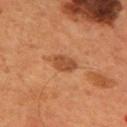<case>
<biopsy_status>not biopsied; imaged during a skin examination</biopsy_status>
<lesion_size>
  <long_diameter_mm_approx>3.5</long_diameter_mm_approx>
</lesion_size>
<patient>
  <sex>male</sex>
  <age_approx>55</age_approx>
</patient>
<image>
  <source>total-body photography crop</source>
  <field_of_view_mm>15</field_of_view_mm>
</image>
<lighting>cross-polarized</lighting>
<site>upper back</site>
</case>